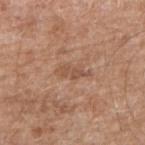| feature | finding |
|---|---|
| notes | total-body-photography surveillance lesion; no biopsy |
| TBP lesion metrics | an area of roughly 3.5 mm², an outline eccentricity of about 0.9 (0 = round, 1 = elongated), and a shape-asymmetry score of about 0.4 (0 = symmetric); a mean CIELAB color near L≈52 a*≈20 b*≈31, roughly 8 lightness units darker than nearby skin, and a normalized border contrast of about 5.5 |
| patient | male, aged 58 to 62 |
| body site | the right upper arm |
| image source | ~15 mm tile from a whole-body skin photo |
| tile lighting | white-light |